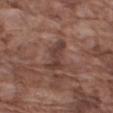Impression: The lesion was tiled from a total-body skin photograph and was not biopsied. Image and clinical context: The lesion is located on the arm. A 15 mm close-up extracted from a 3D total-body photography capture. Automated image analysis of the tile measured a within-lesion color-variation index near 2.5/10 and a peripheral color-asymmetry measure near 1. The software also gave a nevus-likeness score of about 0/100. The lesion's longest dimension is about 4.5 mm. This is a white-light tile. The patient is a male aged around 75.